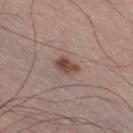Impression:
Captured during whole-body skin photography for melanoma surveillance; the lesion was not biopsied.
Acquisition and patient details:
An algorithmic analysis of the crop reported a footprint of about 4.5 mm² and an outline eccentricity of about 0.8 (0 = round, 1 = elongated). The software also gave a mean CIELAB color near L≈46 a*≈19 b*≈24 and about 12 CIELAB-L* units darker than the surrounding skin. A 15 mm close-up tile from a total-body photography series done for melanoma screening. On the leg. A male subject, aged 53 to 57.